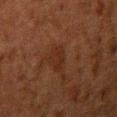notes — catalogued during a skin exam; not biopsied
image — total-body-photography crop, ~15 mm field of view
subject — male, aged 58–62
location — the right upper arm
lesion diameter — ~2.5 mm (longest diameter)
tile lighting — cross-polarized